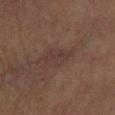biopsy status: catalogued during a skin exam; not biopsied | lesion size: ≈3.5 mm | TBP lesion metrics: a mean CIELAB color near L≈33 a*≈15 b*≈20 and a lesion-to-skin contrast of about 4.5 (normalized; higher = more distinct); a border-irregularity index near 3/10, internal color variation of about 1.5 on a 0–10 scale, and radial color variation of about 0.5; a detector confidence of about 95 out of 100 that the crop contains a lesion | patient: female, in their mid- to late 60s | site: the right lower leg | image source: total-body-photography crop, ~15 mm field of view | lighting: cross-polarized.